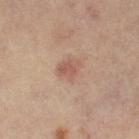| field | value |
|---|---|
| follow-up | no biopsy performed (imaged during a skin exam) |
| patient | female, aged approximately 65 |
| image | total-body-photography crop, ~15 mm field of view |
| lesion size | ~2.5 mm (longest diameter) |
| tile lighting | cross-polarized illumination |
| anatomic site | the right leg |
| automated lesion analysis | a lesion area of about 5.5 mm², an eccentricity of roughly 0.45, and a shape-asymmetry score of about 0.25 (0 = symmetric); an average lesion color of about L≈54 a*≈20 b*≈26 (CIELAB) and roughly 8 lightness units darker than nearby skin; a border-irregularity rating of about 2.5/10, internal color variation of about 2.5 on a 0–10 scale, and radial color variation of about 1 |